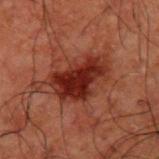{"biopsy_status": "not biopsied; imaged during a skin examination", "automated_metrics": {"lesion_detection_confidence_0_100": 100}, "patient": {"sex": "male", "age_approx": 50}, "lighting": "cross-polarized", "lesion_size": {"long_diameter_mm_approx": 6.0}, "site": "upper back", "image": {"source": "total-body photography crop", "field_of_view_mm": 15}}